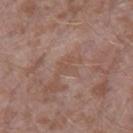No biopsy was performed on this lesion — it was imaged during a full skin examination and was not determined to be concerning. Cropped from a whole-body photographic skin survey; the tile spans about 15 mm. The lesion is on the leg. About 2.5 mm across. The subject is a male about 45 years old.